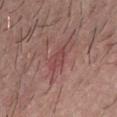notes = no biopsy performed (imaged during a skin exam) | illumination = white-light illumination | image source = 15 mm crop, total-body photography | subject = male, aged approximately 30 | size = ~3 mm (longest diameter) | automated lesion analysis = about 7 CIELAB-L* units darker than the surrounding skin; a nevus-likeness score of about 0/100 and lesion-presence confidence of about 80/100.